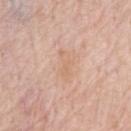biopsy status = catalogued during a skin exam; not biopsied | diameter = about 3.5 mm | automated metrics = an average lesion color of about L≈67 a*≈19 b*≈32 (CIELAB), roughly 5 lightness units darker than nearby skin, and a normalized lesion–skin contrast near 4.5 | anatomic site = the chest | patient = male, aged around 80 | illumination = white-light | acquisition = total-body-photography crop, ~15 mm field of view.